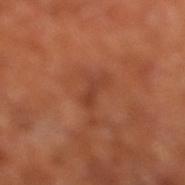| key | value |
|---|---|
| follow-up | no biopsy performed (imaged during a skin exam) |
| subject | in their mid- to late 60s |
| automated lesion analysis | an area of roughly 3.5 mm², an outline eccentricity of about 0.95 (0 = round, 1 = elongated), and a shape-asymmetry score of about 0.35 (0 = symmetric); border irregularity of about 5 on a 0–10 scale, a within-lesion color-variation index near 0/10, and a peripheral color-asymmetry measure near 0; a classifier nevus-likeness of about 0/100 and a lesion-detection confidence of about 85/100 |
| anatomic site | the right lower leg |
| image source | ~15 mm crop, total-body skin-cancer survey |
| size | about 3.5 mm |